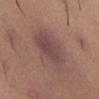A female subject, about 40 years old. Cropped from a total-body skin-imaging series; the visible field is about 15 mm. On the front of the torso.